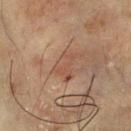Clinical impression:
Recorded during total-body skin imaging; not selected for excision or biopsy.
Context:
A 15 mm crop from a total-body photograph taken for skin-cancer surveillance. A male patient in their 70s. Located on the chest. The lesion's longest dimension is about 3.5 mm.